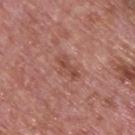Impression:
Part of a total-body skin-imaging series; this lesion was reviewed on a skin check and was not flagged for biopsy.